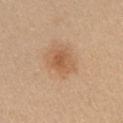follow-up=total-body-photography surveillance lesion; no biopsy | location=the left upper arm | imaging modality=total-body-photography crop, ~15 mm field of view | subject=female, aged 28 to 32 | lesion diameter=about 3 mm | automated metrics=roughly 9 lightness units darker than nearby skin; an automated nevus-likeness rating near 55 out of 100 and a lesion-detection confidence of about 100/100.